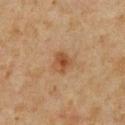The lesion was photographed on a routine skin check and not biopsied; there is no pathology result. A male subject aged 58–62. A 15 mm crop from a total-body photograph taken for skin-cancer surveillance. Captured under cross-polarized illumination. Automated tile analysis of the lesion measured a footprint of about 4.5 mm², an outline eccentricity of about 0.5 (0 = round, 1 = elongated), and a shape-asymmetry score of about 0.25 (0 = symmetric). And it measured an automated nevus-likeness rating near 65 out of 100. The lesion is located on the chest. Measured at roughly 2.5 mm in maximum diameter.